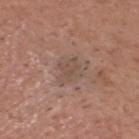{"biopsy_status": "not biopsied; imaged during a skin examination", "image": {"source": "total-body photography crop", "field_of_view_mm": 15}, "lesion_size": {"long_diameter_mm_approx": 3.5}, "patient": {"sex": "male", "age_approx": 35}, "automated_metrics": {"shape_asymmetry": 0.35, "border_irregularity_0_10": 4.0, "color_variation_0_10": 2.5, "nevus_likeness_0_100": 0, "lesion_detection_confidence_0_100": 100}, "site": "head or neck"}